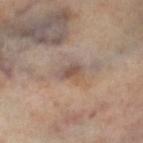This lesion was catalogued during total-body skin photography and was not selected for biopsy.
The lesion-visualizer software estimated a shape eccentricity near 0.8 and two-axis asymmetry of about 0.3. It also reported lesion-presence confidence of about 75/100.
The patient is a female aged 48–52.
The lesion's longest dimension is about 3 mm.
This image is a 15 mm lesion crop taken from a total-body photograph.
From the left lower leg.